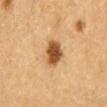Assessment: Part of a total-body skin-imaging series; this lesion was reviewed on a skin check and was not flagged for biopsy. Image and clinical context: A male subject, about 85 years old or older. On the mid back. A 15 mm close-up tile from a total-body photography series done for melanoma screening. Longest diameter approximately 3 mm.